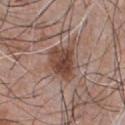{"biopsy_status": "not biopsied; imaged during a skin examination", "automated_metrics": {"area_mm2_approx": 12.0, "eccentricity": 0.65, "cielab_L": 44, "cielab_a": 19, "cielab_b": 25, "vs_skin_darker_L": 12.0, "vs_skin_contrast_norm": 9.5, "peripheral_color_asymmetry": 1.5, "nevus_likeness_0_100": 75}, "lighting": "white-light", "image": {"source": "total-body photography crop", "field_of_view_mm": 15}, "site": "chest", "lesion_size": {"long_diameter_mm_approx": 4.5}, "patient": {"sex": "male", "age_approx": 45}}